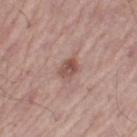Impression:
Imaged during a routine full-body skin examination; the lesion was not biopsied and no histopathology is available.
Context:
Captured under white-light illumination. The recorded lesion diameter is about 2.5 mm. The subject is a male in their mid- to late 50s. The lesion is located on the left thigh. A close-up tile cropped from a whole-body skin photograph, about 15 mm across. An algorithmic analysis of the crop reported a border-irregularity rating of about 2.5/10, internal color variation of about 4.5 on a 0–10 scale, and a peripheral color-asymmetry measure near 1.5.